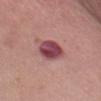Q: Was a biopsy performed?
A: catalogued during a skin exam; not biopsied
Q: What is the lesion's diameter?
A: ~4 mm (longest diameter)
Q: What is the anatomic site?
A: the chest
Q: Automated lesion metrics?
A: a footprint of about 9 mm², an outline eccentricity of about 0.75 (0 = round, 1 = elongated), and two-axis asymmetry of about 0.15; a mean CIELAB color near L≈45 a*≈30 b*≈17, about 16 CIELAB-L* units darker than the surrounding skin, and a lesion-to-skin contrast of about 12 (normalized; higher = more distinct); a within-lesion color-variation index near 6/10 and a peripheral color-asymmetry measure near 2; lesion-presence confidence of about 100/100
Q: Patient demographics?
A: female, aged approximately 65
Q: How was this image acquired?
A: ~15 mm crop, total-body skin-cancer survey